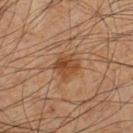biopsy status: catalogued during a skin exam; not biopsied
lesion size: about 3 mm
lighting: cross-polarized
subject: male, aged 58–62
image-analysis metrics: about 9 CIELAB-L* units darker than the surrounding skin and a normalized border contrast of about 8; border irregularity of about 3 on a 0–10 scale, a color-variation rating of about 4.5/10, and radial color variation of about 1.5; a classifier nevus-likeness of about 80/100 and a detector confidence of about 100 out of 100 that the crop contains a lesion
location: the left lower leg
image: ~15 mm crop, total-body skin-cancer survey